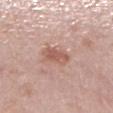tile lighting=white-light illumination; patient=female, aged 48 to 52; diameter=about 3 mm; automated lesion analysis=a nevus-likeness score of about 30/100; site=the right lower leg; acquisition=~15 mm tile from a whole-body skin photo.